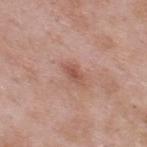biopsy status: catalogued during a skin exam; not biopsied
subject: male, approximately 55 years of age
illumination: white-light
acquisition: ~15 mm crop, total-body skin-cancer survey
location: the upper back
automated lesion analysis: a lesion area of about 3.5 mm² and an eccentricity of roughly 0.85; a detector confidence of about 100 out of 100 that the crop contains a lesion
lesion diameter: ~3 mm (longest diameter)